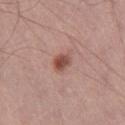Case summary:
* biopsy status · no biopsy performed (imaged during a skin exam)
* patient · male, in their mid- to late 50s
* site · the right thigh
* image · 15 mm crop, total-body photography